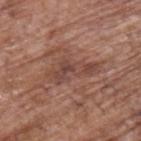Impression:
Part of a total-body skin-imaging series; this lesion was reviewed on a skin check and was not flagged for biopsy.
Background:
Cropped from a whole-body photographic skin survey; the tile spans about 15 mm. The lesion is on the upper back. The subject is a male roughly 70 years of age.